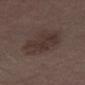Part of a total-body skin-imaging series; this lesion was reviewed on a skin check and was not flagged for biopsy.
A male patient, in their 70s.
A roughly 15 mm field-of-view crop from a total-body skin photograph.
Longest diameter approximately 5.5 mm.
The lesion is located on the right lower leg.
The lesion-visualizer software estimated an area of roughly 18 mm² and two-axis asymmetry of about 0.25. And it measured an average lesion color of about L≈32 a*≈14 b*≈18 (CIELAB), roughly 7 lightness units darker than nearby skin, and a normalized border contrast of about 6.5. It also reported internal color variation of about 3 on a 0–10 scale.